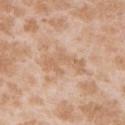This lesion was catalogued during total-body skin photography and was not selected for biopsy. Captured under white-light illumination. The lesion is located on the right upper arm. A female patient approximately 25 years of age. Automated image analysis of the tile measured two-axis asymmetry of about 0.4. The analysis additionally found a mean CIELAB color near L≈64 a*≈18 b*≈33, roughly 7 lightness units darker than nearby skin, and a lesion-to-skin contrast of about 5 (normalized; higher = more distinct). And it measured lesion-presence confidence of about 95/100. Cropped from a total-body skin-imaging series; the visible field is about 15 mm. The lesion's longest dimension is about 5 mm.